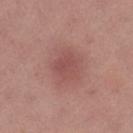Captured during whole-body skin photography for melanoma surveillance; the lesion was not biopsied. Located on the left thigh. A lesion tile, about 15 mm wide, cut from a 3D total-body photograph. A female patient, about 55 years old.